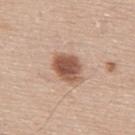The lesion was tiled from a total-body skin photograph and was not biopsied. The lesion-visualizer software estimated a shape eccentricity near 0.65 and two-axis asymmetry of about 0.2. And it measured a lesion color around L≈54 a*≈21 b*≈29 in CIELAB. The analysis additionally found a color-variation rating of about 4/10 and radial color variation of about 1. It also reported a nevus-likeness score of about 100/100 and a detector confidence of about 100 out of 100 that the crop contains a lesion. This is a white-light tile. A roughly 15 mm field-of-view crop from a total-body skin photograph. The lesion is located on the upper back. The lesion's longest dimension is about 4 mm. The patient is a male approximately 60 years of age.